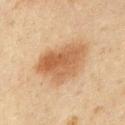Clinical impression:
Part of a total-body skin-imaging series; this lesion was reviewed on a skin check and was not flagged for biopsy.
Clinical summary:
The lesion is located on the chest. Cropped from a whole-body photographic skin survey; the tile spans about 15 mm. The total-body-photography lesion software estimated a within-lesion color-variation index near 5/10 and peripheral color asymmetry of about 1.5. The lesion's longest dimension is about 6.5 mm. A male patient, aged 58 to 62. This is a cross-polarized tile.